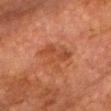Impression:
Imaged during a routine full-body skin examination; the lesion was not biopsied and no histopathology is available.
Background:
A lesion tile, about 15 mm wide, cut from a 3D total-body photograph. From the chest. The total-body-photography lesion software estimated a lesion color around L≈40 a*≈24 b*≈32 in CIELAB, roughly 6 lightness units darker than nearby skin, and a lesion-to-skin contrast of about 6 (normalized; higher = more distinct). The recorded lesion diameter is about 4 mm. A male patient, aged 73–77.